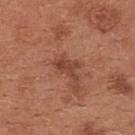This lesion was catalogued during total-body skin photography and was not selected for biopsy.
A female subject, roughly 35 years of age.
This is a white-light tile.
A roughly 15 mm field-of-view crop from a total-body skin photograph.
The lesion is on the front of the torso.